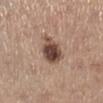{"biopsy_status": "not biopsied; imaged during a skin examination", "patient": {"sex": "female", "age_approx": 65}, "site": "leg", "image": {"source": "total-body photography crop", "field_of_view_mm": 15}, "automated_metrics": {"area_mm2_approx": 9.5, "eccentricity": 0.5, "shape_asymmetry": 0.2, "cielab_L": 46, "cielab_a": 18, "cielab_b": 24, "color_variation_0_10": 6.5, "peripheral_color_asymmetry": 2.0, "nevus_likeness_0_100": 90, "lesion_detection_confidence_0_100": 100}, "lesion_size": {"long_diameter_mm_approx": 3.5}, "lighting": "white-light"}